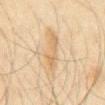This is a cross-polarized tile.
The recorded lesion diameter is about 5 mm.
A male subject aged around 65.
A 15 mm crop from a total-body photograph taken for skin-cancer surveillance.
On the front of the torso.